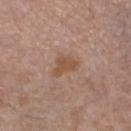– biopsy status — imaged on a skin check; not biopsied
– size — about 3 mm
– TBP lesion metrics — an area of roughly 5 mm², an outline eccentricity of about 0.75 (0 = round, 1 = elongated), and two-axis asymmetry of about 0.5; a border-irregularity rating of about 4.5/10 and a peripheral color-asymmetry measure near 0.5; a classifier nevus-likeness of about 5/100 and lesion-presence confidence of about 100/100
– acquisition — 15 mm crop, total-body photography
– site — the left lower leg
– patient — female, aged 53 to 57